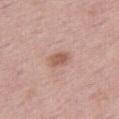{
  "patient": {
    "sex": "male",
    "age_approx": 55
  },
  "site": "right thigh",
  "lesion_size": {
    "long_diameter_mm_approx": 2.5
  },
  "lighting": "white-light",
  "image": {
    "source": "total-body photography crop",
    "field_of_view_mm": 15
  }
}